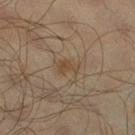| feature | finding |
|---|---|
| follow-up | total-body-photography surveillance lesion; no biopsy |
| image source | ~15 mm crop, total-body skin-cancer survey |
| location | the right lower leg |
| lesion size | about 2.5 mm |
| patient | male, aged approximately 65 |